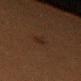<record>
<biopsy_status>not biopsied; imaged during a skin examination</biopsy_status>
<patient>
  <sex>female</sex>
  <age_approx>40</age_approx>
</patient>
<image>
  <source>total-body photography crop</source>
  <field_of_view_mm>15</field_of_view_mm>
</image>
<site>left thigh</site>
<lesion_size>
  <long_diameter_mm_approx>2.5</long_diameter_mm_approx>
</lesion_size>
</record>